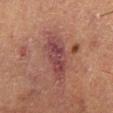Assessment:
This lesion was catalogued during total-body skin photography and was not selected for biopsy.
Clinical summary:
Located on the right lower leg. Approximately 7 mm at its widest. A lesion tile, about 15 mm wide, cut from a 3D total-body photograph. A male subject, aged around 55. This is a cross-polarized tile. An algorithmic analysis of the crop reported an area of roughly 14 mm², a shape eccentricity near 0.9, and a symmetry-axis asymmetry near 0.2. It also reported a mean CIELAB color near L≈38 a*≈22 b*≈19, roughly 8 lightness units darker than nearby skin, and a lesion-to-skin contrast of about 8 (normalized; higher = more distinct). The software also gave a classifier nevus-likeness of about 0/100 and a detector confidence of about 90 out of 100 that the crop contains a lesion.